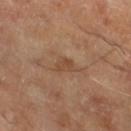{"biopsy_status": "not biopsied; imaged during a skin examination", "patient": {"sex": "male", "age_approx": 65}, "image": {"source": "total-body photography crop", "field_of_view_mm": 15}, "lesion_size": {"long_diameter_mm_approx": 2.5}, "lighting": "cross-polarized", "site": "left lower leg"}